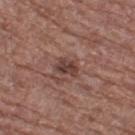size — about 3 mm | image-analysis metrics — a lesion-to-skin contrast of about 8.5 (normalized; higher = more distinct); a border-irregularity rating of about 3/10, a within-lesion color-variation index near 3/10, and peripheral color asymmetry of about 1; a classifier nevus-likeness of about 0/100 and a detector confidence of about 100 out of 100 that the crop contains a lesion | anatomic site — the left thigh | imaging modality — total-body-photography crop, ~15 mm field of view | tile lighting — white-light illumination | patient — female, aged around 75.